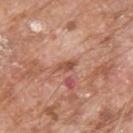A region of skin cropped from a whole-body photographic capture, roughly 15 mm wide. A male patient, about 80 years old. On the upper back.